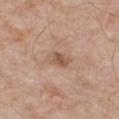| feature | finding |
|---|---|
| workup | catalogued during a skin exam; not biopsied |
| lighting | white-light illumination |
| automated lesion analysis | a mean CIELAB color near L≈54 a*≈19 b*≈30, roughly 10 lightness units darker than nearby skin, and a normalized lesion–skin contrast near 7 |
| imaging modality | ~15 mm crop, total-body skin-cancer survey |
| patient | male, in their mid- to late 60s |
| anatomic site | the chest |
| diameter | ≈2.5 mm |